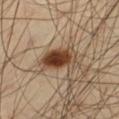{
  "biopsy_status": "not biopsied; imaged during a skin examination",
  "lighting": "cross-polarized",
  "site": "left thigh",
  "image": {
    "source": "total-body photography crop",
    "field_of_view_mm": 15
  },
  "patient": {
    "sex": "male",
    "age_approx": 40
  },
  "lesion_size": {
    "long_diameter_mm_approx": 4.5
  }
}